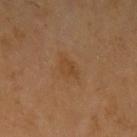image:
  source: total-body photography crop
  field_of_view_mm: 15
site: left upper arm
patient:
  sex: male
  age_approx: 70